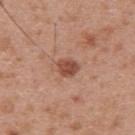Clinical impression: No biopsy was performed on this lesion — it was imaged during a full skin examination and was not determined to be concerning. Image and clinical context: The subject is a male roughly 30 years of age. The lesion is located on the upper back. The lesion's longest dimension is about 2.5 mm. A 15 mm close-up extracted from a 3D total-body photography capture.